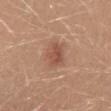<lesion>
<biopsy_status>not biopsied; imaged during a skin examination</biopsy_status>
<site>mid back</site>
<patient>
  <sex>female</sex>
  <age_approx>30</age_approx>
</patient>
<image>
  <source>total-body photography crop</source>
  <field_of_view_mm>15</field_of_view_mm>
</image>
</lesion>